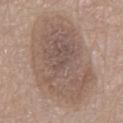<case>
  <biopsy_status>not biopsied; imaged during a skin examination</biopsy_status>
  <site>mid back</site>
  <image>
    <source>total-body photography crop</source>
    <field_of_view_mm>15</field_of_view_mm>
  </image>
  <automated_metrics>
    <border_irregularity_0_10>2.5</border_irregularity_0_10>
    <color_variation_0_10>4.5</color_variation_0_10>
  </automated_metrics>
  <lighting>white-light</lighting>
  <patient>
    <sex>male</sex>
    <age_approx>55</age_approx>
  </patient>
  <lesion_size>
    <long_diameter_mm_approx>12.0</long_diameter_mm_approx>
  </lesion_size>
</case>